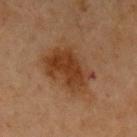{
  "biopsy_status": "not biopsied; imaged during a skin examination",
  "site": "left upper arm",
  "patient": {
    "sex": "female",
    "age_approx": 70
  },
  "image": {
    "source": "total-body photography crop",
    "field_of_view_mm": 15
  },
  "automated_metrics": {
    "cielab_L": 34,
    "cielab_a": 19,
    "cielab_b": 30,
    "vs_skin_darker_L": 8.0,
    "vs_skin_contrast_norm": 8.0,
    "color_variation_0_10": 4.5
  },
  "lighting": "cross-polarized",
  "lesion_size": {
    "long_diameter_mm_approx": 6.5
  }
}